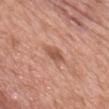Part of a total-body skin-imaging series; this lesion was reviewed on a skin check and was not flagged for biopsy.
From the chest.
A male patient, in their mid- to late 50s.
This image is a 15 mm lesion crop taken from a total-body photograph.